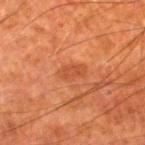Findings:
- workup: imaged on a skin check; not biopsied
- automated lesion analysis: a footprint of about 4.5 mm², an eccentricity of roughly 0.8, and a symmetry-axis asymmetry near 0.25; a border-irregularity index near 2.5/10, internal color variation of about 2 on a 0–10 scale, and peripheral color asymmetry of about 0.5
- body site: the right lower leg
- illumination: cross-polarized illumination
- subject: male, approximately 80 years of age
- acquisition: ~15 mm tile from a whole-body skin photo
- lesion diameter: ~3 mm (longest diameter)